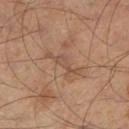Case summary:
* workup: imaged on a skin check; not biopsied
* site: the leg
* imaging modality: ~15 mm crop, total-body skin-cancer survey
* patient: male, aged 53 to 57
* illumination: cross-polarized